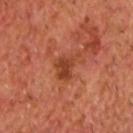Context:
A male subject approximately 65 years of age. A close-up tile cropped from a whole-body skin photograph, about 15 mm across. Captured under cross-polarized illumination.